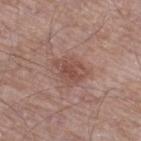Imaged during a routine full-body skin examination; the lesion was not biopsied and no histopathology is available. Measured at roughly 3.5 mm in maximum diameter. On the leg. Cropped from a whole-body photographic skin survey; the tile spans about 15 mm. Automated image analysis of the tile measured lesion-presence confidence of about 100/100. A male patient aged 68 to 72.